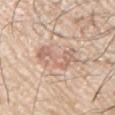Assessment: The lesion was photographed on a routine skin check and not biopsied; there is no pathology result. Clinical summary: A male patient aged 63 to 67. Imaged with white-light lighting. A region of skin cropped from a whole-body photographic capture, roughly 15 mm wide. Longest diameter approximately 5.5 mm. The lesion is on the arm.